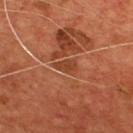Assessment:
Captured during whole-body skin photography for melanoma surveillance; the lesion was not biopsied.
Clinical summary:
An algorithmic analysis of the crop reported lesion-presence confidence of about 90/100. Imaged with cross-polarized lighting. A lesion tile, about 15 mm wide, cut from a 3D total-body photograph. About 3 mm across. The subject is a male in their mid-50s. The lesion is on the front of the torso.